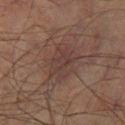anatomic site: the right lower leg
image: ~15 mm crop, total-body skin-cancer survey
subject: male, approximately 70 years of age